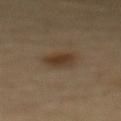<case>
  <biopsy_status>not biopsied; imaged during a skin examination</biopsy_status>
  <image>
    <source>total-body photography crop</source>
    <field_of_view_mm>15</field_of_view_mm>
  </image>
  <automated_metrics>
    <cielab_L>36</cielab_L>
    <cielab_a>13</cielab_a>
    <cielab_b>27</cielab_b>
    <vs_skin_darker_L>8.0</vs_skin_darker_L>
    <lesion_detection_confidence_0_100>100</lesion_detection_confidence_0_100>
  </automated_metrics>
  <lesion_size>
    <long_diameter_mm_approx>3.0</long_diameter_mm_approx>
  </lesion_size>
  <site>abdomen</site>
  <patient>
    <sex>male</sex>
    <age_approx>65</age_approx>
  </patient>
  <lighting>cross-polarized</lighting>
</case>